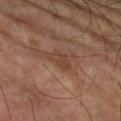Imaged during a routine full-body skin examination; the lesion was not biopsied and no histopathology is available.
The lesion's longest dimension is about 3 mm.
A roughly 15 mm field-of-view crop from a total-body skin photograph.
Automated image analysis of the tile measured about 6 CIELAB-L* units darker than the surrounding skin and a lesion-to-skin contrast of about 5.5 (normalized; higher = more distinct). The software also gave a border-irregularity rating of about 3/10, a color-variation rating of about 3.5/10, and radial color variation of about 1. The software also gave a classifier nevus-likeness of about 0/100.
On the left upper arm.
A male patient aged 58 to 62.